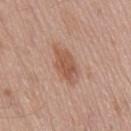Q: Is there a histopathology result?
A: no biopsy performed (imaged during a skin exam)
Q: What are the patient's age and sex?
A: male, about 75 years old
Q: What is the anatomic site?
A: the back
Q: What kind of image is this?
A: 15 mm crop, total-body photography
Q: How large is the lesion?
A: ≈5 mm
Q: Automated lesion metrics?
A: a footprint of about 10 mm² and an outline eccentricity of about 0.85 (0 = round, 1 = elongated); a border-irregularity index near 3/10 and a within-lesion color-variation index near 3.5/10